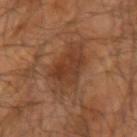Captured during whole-body skin photography for melanoma surveillance; the lesion was not biopsied. Located on the right upper arm. Automated tile analysis of the lesion measured an area of roughly 16 mm², an outline eccentricity of about 0.7 (0 = round, 1 = elongated), and a shape-asymmetry score of about 0.2 (0 = symmetric). It also reported a nevus-likeness score of about 20/100 and lesion-presence confidence of about 100/100. A male patient, roughly 65 years of age. This is a cross-polarized tile. A 15 mm close-up extracted from a 3D total-body photography capture.